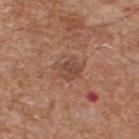* follow-up: no biopsy performed (imaged during a skin exam)
* site: the upper back
* illumination: white-light illumination
* image source: ~15 mm crop, total-body skin-cancer survey
* image-analysis metrics: internal color variation of about 4 on a 0–10 scale and radial color variation of about 1.5; a nevus-likeness score of about 0/100 and a lesion-detection confidence of about 100/100
* subject: male, roughly 65 years of age